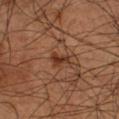Captured during whole-body skin photography for melanoma surveillance; the lesion was not biopsied.
The lesion is located on the arm.
A lesion tile, about 15 mm wide, cut from a 3D total-body photograph.
The tile uses cross-polarized illumination.
The lesion's longest dimension is about 2.5 mm.
A male patient, aged 53 to 57.